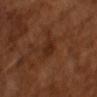Notes:
- biopsy status · catalogued during a skin exam; not biopsied
- diameter · ≈3 mm
- image · ~15 mm crop, total-body skin-cancer survey
- patient · male, roughly 65 years of age